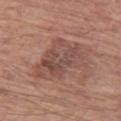Q: Was this lesion biopsied?
A: catalogued during a skin exam; not biopsied
Q: Where on the body is the lesion?
A: the left thigh
Q: Automated lesion metrics?
A: a footprint of about 22 mm², an outline eccentricity of about 0.75 (0 = round, 1 = elongated), and two-axis asymmetry of about 0.3; a lesion color around L≈48 a*≈20 b*≈24 in CIELAB, a lesion–skin lightness drop of about 8, and a lesion-to-skin contrast of about 6.5 (normalized; higher = more distinct); a border-irregularity rating of about 4.5/10, a within-lesion color-variation index near 5/10, and a peripheral color-asymmetry measure near 1.5; a classifier nevus-likeness of about 0/100 and a lesion-detection confidence of about 100/100
Q: How large is the lesion?
A: ≈7 mm
Q: What kind of image is this?
A: 15 mm crop, total-body photography
Q: What are the patient's age and sex?
A: male, in their mid- to late 60s
Q: How was the tile lit?
A: white-light illumination